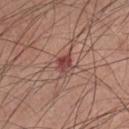Part of a total-body skin-imaging series; this lesion was reviewed on a skin check and was not flagged for biopsy. The recorded lesion diameter is about 3 mm. Captured under white-light illumination. A male subject, aged approximately 25. A close-up tile cropped from a whole-body skin photograph, about 15 mm across. Located on the front of the torso.